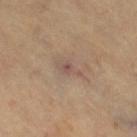biopsy status: total-body-photography surveillance lesion; no biopsy | body site: the right thigh | automated metrics: a border-irregularity index near 4/10, a color-variation rating of about 3/10, and peripheral color asymmetry of about 0.5 | image: ~15 mm tile from a whole-body skin photo | patient: female, roughly 70 years of age | diameter: ~3 mm (longest diameter) | tile lighting: cross-polarized.